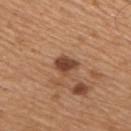| feature | finding |
|---|---|
| workup | imaged on a skin check; not biopsied |
| imaging modality | ~15 mm crop, total-body skin-cancer survey |
| tile lighting | white-light |
| patient | male, in their mid-60s |
| automated lesion analysis | a footprint of about 5 mm² and an eccentricity of roughly 0.6; a lesion color around L≈44 a*≈22 b*≈31 in CIELAB, about 13 CIELAB-L* units darker than the surrounding skin, and a normalized lesion–skin contrast near 9.5; a classifier nevus-likeness of about 85/100 and a lesion-detection confidence of about 100/100 |
| site | the upper back |
| lesion diameter | ~3 mm (longest diameter) |